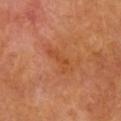Context: A region of skin cropped from a whole-body photographic capture, roughly 15 mm wide. The lesion is on the upper back. The patient is a female aged around 75. The recorded lesion diameter is about 4 mm. This is a cross-polarized tile.